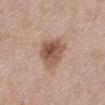{
  "biopsy_status": "not biopsied; imaged during a skin examination",
  "lighting": "white-light",
  "site": "left lower leg",
  "patient": {
    "sex": "female",
    "age_approx": 40
  },
  "image": {
    "source": "total-body photography crop",
    "field_of_view_mm": 15
  }
}